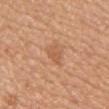<case>
<biopsy_status>not biopsied; imaged during a skin examination</biopsy_status>
<image>
  <source>total-body photography crop</source>
  <field_of_view_mm>15</field_of_view_mm>
</image>
<site>chest</site>
<patient>
  <sex>female</sex>
  <age_approx>40</age_approx>
</patient>
</case>